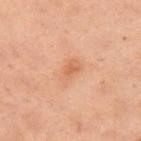Captured during whole-body skin photography for melanoma surveillance; the lesion was not biopsied. Approximately 3 mm at its widest. This is a cross-polarized tile. The total-body-photography lesion software estimated a border-irregularity index near 2/10, a color-variation rating of about 2/10, and radial color variation of about 0.5. A close-up tile cropped from a whole-body skin photograph, about 15 mm across. From the front of the torso. A female subject about 55 years old.